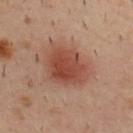Case summary:
- biopsy status · catalogued during a skin exam; not biopsied
- imaging modality · total-body-photography crop, ~15 mm field of view
- patient · male, aged 38 to 42
- automated metrics · a lesion–skin lightness drop of about 11 and a normalized lesion–skin contrast near 8.5
- tile lighting · cross-polarized
- location · the upper back
- lesion diameter · about 5.5 mm